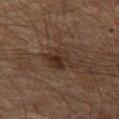Captured during whole-body skin photography for melanoma surveillance; the lesion was not biopsied. Cropped from a whole-body photographic skin survey; the tile spans about 15 mm. The lesion's longest dimension is about 5 mm. The subject is a male about 60 years old. This is a cross-polarized tile. From the leg. The total-body-photography lesion software estimated a footprint of about 8.5 mm², an eccentricity of roughly 0.8, and a shape-asymmetry score of about 0.25 (0 = symmetric). And it measured a border-irregularity index near 3/10.